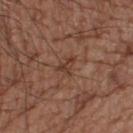{
  "biopsy_status": "not biopsied; imaged during a skin examination",
  "lesion_size": {
    "long_diameter_mm_approx": 3.0
  },
  "patient": {
    "sex": "male",
    "age_approx": 65
  },
  "lighting": "white-light",
  "site": "back",
  "image": {
    "source": "total-body photography crop",
    "field_of_view_mm": 15
  }
}